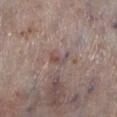Notes:
– notes: catalogued during a skin exam; not biopsied
– lesion size: ≈2.5 mm
– illumination: white-light
– image: ~15 mm crop, total-body skin-cancer survey
– body site: the left lower leg
– patient: male, in their 80s
– TBP lesion metrics: a lesion color around L≈49 a*≈16 b*≈19 in CIELAB and a lesion-to-skin contrast of about 5.5 (normalized; higher = more distinct); a border-irregularity index near 4.5/10, internal color variation of about 1 on a 0–10 scale, and peripheral color asymmetry of about 0